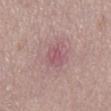Imaged during a routine full-body skin examination; the lesion was not biopsied and no histopathology is available. A lesion tile, about 15 mm wide, cut from a 3D total-body photograph. Captured under white-light illumination. Longest diameter approximately 4 mm. The lesion is on the mid back. Automated tile analysis of the lesion measured a shape eccentricity near 0.85 and a symmetry-axis asymmetry near 0.35. It also reported a mean CIELAB color near L≈54 a*≈24 b*≈16, about 8 CIELAB-L* units darker than the surrounding skin, and a normalized border contrast of about 6. The software also gave a lesion-detection confidence of about 100/100. A male subject, in their mid- to late 50s.